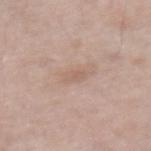Impression:
Captured during whole-body skin photography for melanoma surveillance; the lesion was not biopsied.
Clinical summary:
About 2.5 mm across. Cropped from a whole-body photographic skin survey; the tile spans about 15 mm. A female subject about 65 years old. Captured under white-light illumination. The lesion is on the left leg.